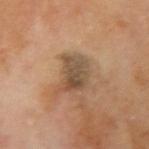The patient is a male aged 68 to 72.
Located on the right upper arm.
A close-up tile cropped from a whole-body skin photograph, about 15 mm across.